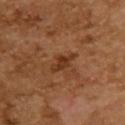<record>
  <biopsy_status>not biopsied; imaged during a skin examination</biopsy_status>
</record>